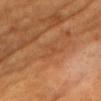Case summary:
– notes — catalogued during a skin exam; not biopsied
– image — ~15 mm tile from a whole-body skin photo
– lesion size — ≈2.5 mm
– subject — female, in their 70s
– automated metrics — a color-variation rating of about 1.5/10; an automated nevus-likeness rating near 0 out of 100
– illumination — cross-polarized
– location — the head or neck